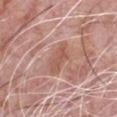biopsy status: imaged on a skin check; not biopsied | illumination: white-light | image: ~15 mm crop, total-body skin-cancer survey | site: the chest | patient: male, aged 48–52 | lesion size: ≈4.5 mm | automated lesion analysis: an outline eccentricity of about 0.9 (0 = round, 1 = elongated) and a symmetry-axis asymmetry near 0.25; about 8 CIELAB-L* units darker than the surrounding skin; lesion-presence confidence of about 50/100.